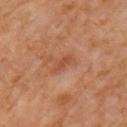Notes:
• location · the left upper arm
• patient · male, aged approximately 55
• acquisition · 15 mm crop, total-body photography
• lesion diameter · ≈3 mm